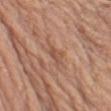Imaged during a routine full-body skin examination; the lesion was not biopsied and no histopathology is available.
A close-up tile cropped from a whole-body skin photograph, about 15 mm across.
From the left upper arm.
Automated tile analysis of the lesion measured a lesion area of about 2.5 mm² and two-axis asymmetry of about 0.35. And it measured an average lesion color of about L≈51 a*≈22 b*≈30 (CIELAB) and a normalized border contrast of about 6.
A male subject roughly 60 years of age.
The lesion's longest dimension is about 2.5 mm.
Captured under white-light illumination.